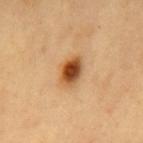{
  "biopsy_status": "not biopsied; imaged during a skin examination",
  "patient": {
    "sex": "male",
    "age_approx": 60
  },
  "automated_metrics": {
    "area_mm2_approx": 7.0,
    "eccentricity": 0.75,
    "shape_asymmetry": 0.15,
    "border_irregularity_0_10": 1.5,
    "color_variation_0_10": 7.5,
    "peripheral_color_asymmetry": 2.0,
    "nevus_likeness_0_100": 100,
    "lesion_detection_confidence_0_100": 100
  },
  "image": {
    "source": "total-body photography crop",
    "field_of_view_mm": 15
  },
  "site": "mid back"
}